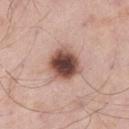Notes:
* workup: total-body-photography surveillance lesion; no biopsy
* imaging modality: ~15 mm tile from a whole-body skin photo
* patient: male, aged 53–57
* site: the leg